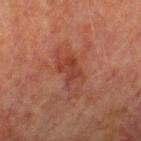Case summary:
- follow-up · imaged on a skin check; not biopsied
- patient · male, in their mid-70s
- image-analysis metrics · a footprint of about 5.5 mm², an outline eccentricity of about 0.65 (0 = round, 1 = elongated), and a shape-asymmetry score of about 0.35 (0 = symmetric)
- tile lighting · cross-polarized illumination
- diameter · ≈3 mm
- image source · 15 mm crop, total-body photography
- location · the left forearm